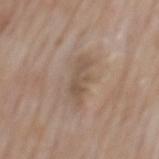Background:
Located on the mid back. The total-body-photography lesion software estimated an area of roughly 6 mm², a shape eccentricity near 0.9, and a shape-asymmetry score of about 0.45 (0 = symmetric). The software also gave a mean CIELAB color near L≈52 a*≈14 b*≈26 and roughly 8 lightness units darker than nearby skin. It also reported an automated nevus-likeness rating near 0 out of 100 and lesion-presence confidence of about 100/100. This image is a 15 mm lesion crop taken from a total-body photograph. A male patient about 60 years old. The lesion's longest dimension is about 4 mm. Captured under white-light illumination.